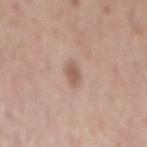Recorded during total-body skin imaging; not selected for excision or biopsy.
A male patient roughly 70 years of age.
This image is a 15 mm lesion crop taken from a total-body photograph.
Imaged with white-light lighting.
The lesion's longest dimension is about 3 mm.
The lesion is on the mid back.